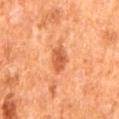Clinical impression:
The lesion was photographed on a routine skin check and not biopsied; there is no pathology result.
Image and clinical context:
Cropped from a whole-body photographic skin survey; the tile spans about 15 mm. The subject is a male approximately 65 years of age. An algorithmic analysis of the crop reported a footprint of about 6 mm², an eccentricity of roughly 0.85, and two-axis asymmetry of about 0.2. The software also gave a classifier nevus-likeness of about 85/100. The lesion is on the mid back. Imaged with cross-polarized lighting.